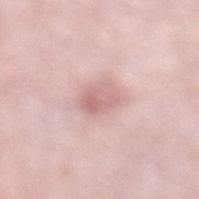notes: catalogued during a skin exam; not biopsied | body site: the leg | acquisition: ~15 mm tile from a whole-body skin photo | subject: female, aged 38–42.